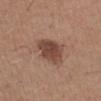follow-up: catalogued during a skin exam; not biopsied
anatomic site: the left upper arm
tile lighting: white-light illumination
patient: male, approximately 50 years of age
lesion size: ≈5 mm
image source: total-body-photography crop, ~15 mm field of view